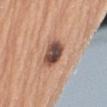Notes:
- follow-up · imaged on a skin check; not biopsied
- patient · female, aged around 65
- image · ~15 mm tile from a whole-body skin photo
- site · the right upper arm